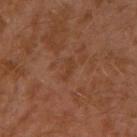Recorded during total-body skin imaging; not selected for excision or biopsy.
The lesion's longest dimension is about 2.5 mm.
The lesion is located on the left arm.
Automated tile analysis of the lesion measured a mean CIELAB color near L≈37 a*≈21 b*≈31, roughly 4 lightness units darker than nearby skin, and a normalized lesion–skin contrast near 4.5. It also reported a classifier nevus-likeness of about 0/100.
This is a cross-polarized tile.
A male patient, aged 28 to 32.
A 15 mm close-up extracted from a 3D total-body photography capture.